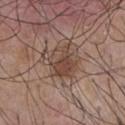Q: Is there a histopathology result?
A: total-body-photography surveillance lesion; no biopsy
Q: Where on the body is the lesion?
A: the chest
Q: Who is the patient?
A: male, approximately 55 years of age
Q: Illumination type?
A: white-light illumination
Q: What did automated image analysis measure?
A: a border-irregularity index near 2.5/10 and radial color variation of about 2; a nevus-likeness score of about 50/100 and a lesion-detection confidence of about 100/100
Q: How was this image acquired?
A: ~15 mm crop, total-body skin-cancer survey